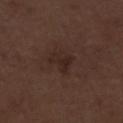Impression:
Recorded during total-body skin imaging; not selected for excision or biopsy.
Clinical summary:
A 15 mm close-up extracted from a 3D total-body photography capture. The lesion is on the right lower leg. The lesion's longest dimension is about 3 mm. The lesion-visualizer software estimated a lesion area of about 6 mm² and two-axis asymmetry of about 0.45. The software also gave a mean CIELAB color near L≈24 a*≈15 b*≈19 and a normalized lesion–skin contrast near 6. The software also gave a classifier nevus-likeness of about 5/100 and a detector confidence of about 100 out of 100 that the crop contains a lesion. Captured under white-light illumination. A male patient approximately 70 years of age.